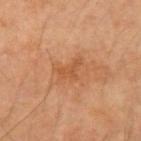No biopsy was performed on this lesion — it was imaged during a full skin examination and was not determined to be concerning.
The lesion-visualizer software estimated a footprint of about 4.5 mm² and two-axis asymmetry of about 0.5. And it measured a lesion color around L≈51 a*≈24 b*≈38 in CIELAB, a lesion–skin lightness drop of about 7, and a normalized lesion–skin contrast near 5.5. And it measured a border-irregularity index near 5.5/10, internal color variation of about 1 on a 0–10 scale, and peripheral color asymmetry of about 0.5. The software also gave an automated nevus-likeness rating near 0 out of 100 and lesion-presence confidence of about 100/100.
On the left upper arm.
Imaged with cross-polarized lighting.
A male subject in their mid- to late 60s.
About 3 mm across.
Cropped from a total-body skin-imaging series; the visible field is about 15 mm.